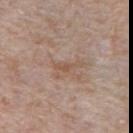biopsy_status: not biopsied; imaged during a skin examination
lighting: white-light
site: right upper arm
patient:
  sex: male
  age_approx: 70
automated_metrics:
  cielab_L: 53
  cielab_a: 18
  cielab_b: 28
  vs_skin_contrast_norm: 6.0
  nevus_likeness_0_100: 0
  lesion_detection_confidence_0_100: 100
image:
  source: total-body photography crop
  field_of_view_mm: 15